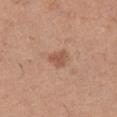• biopsy status: total-body-photography surveillance lesion; no biopsy
• location: the left thigh
• image: ~15 mm crop, total-body skin-cancer survey
• diameter: about 2.5 mm
• tile lighting: white-light illumination
• automated lesion analysis: a lesion color around L≈54 a*≈21 b*≈31 in CIELAB and about 9 CIELAB-L* units darker than the surrounding skin; border irregularity of about 4 on a 0–10 scale, internal color variation of about 0.5 on a 0–10 scale, and radial color variation of about 0; a detector confidence of about 100 out of 100 that the crop contains a lesion
• patient: female, roughly 40 years of age